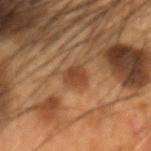<lesion>
  <biopsy_status>not biopsied; imaged during a skin examination</biopsy_status>
  <site>head or neck</site>
  <image>
    <source>total-body photography crop</source>
    <field_of_view_mm>15</field_of_view_mm>
  </image>
  <patient>
    <sex>male</sex>
    <age_approx>55</age_approx>
  </patient>
</lesion>